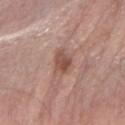Assessment: Recorded during total-body skin imaging; not selected for excision or biopsy. Image and clinical context: A region of skin cropped from a whole-body photographic capture, roughly 15 mm wide. A male subject, in their 60s. On the right forearm.